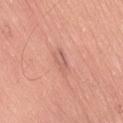Q: Was a biopsy performed?
A: total-body-photography surveillance lesion; no biopsy
Q: What did automated image analysis measure?
A: a lesion area of about 3 mm², a shape eccentricity near 0.85, and two-axis asymmetry of about 0.3; an average lesion color of about L≈61 a*≈26 b*≈28 (CIELAB)
Q: What lighting was used for the tile?
A: white-light illumination
Q: How large is the lesion?
A: ~2.5 mm (longest diameter)
Q: Lesion location?
A: the right thigh
Q: How was this image acquired?
A: total-body-photography crop, ~15 mm field of view
Q: Patient demographics?
A: male, approximately 50 years of age